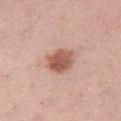Case summary:
* follow-up: no biopsy performed (imaged during a skin exam)
* patient: female, about 40 years old
* image source: 15 mm crop, total-body photography
* lesion size: ≈3.5 mm
* lighting: white-light illumination
* site: the left thigh